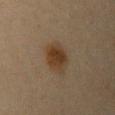No biopsy was performed on this lesion — it was imaged during a full skin examination and was not determined to be concerning.
The tile uses cross-polarized illumination.
From the left upper arm.
A 15 mm crop from a total-body photograph taken for skin-cancer surveillance.
The subject is a female in their mid-40s.